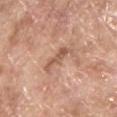Case summary:
* follow-up · catalogued during a skin exam; not biopsied
* body site · the upper back
* illumination · white-light
* subject · male, approximately 65 years of age
* imaging modality · total-body-photography crop, ~15 mm field of view